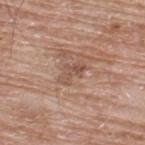workup: no biopsy performed (imaged during a skin exam) | subject: male, aged 63 to 67 | lesion size: ~3.5 mm (longest diameter) | image-analysis metrics: an outline eccentricity of about 0.85 (0 = round, 1 = elongated) and a symmetry-axis asymmetry near 0.4; an average lesion color of about L≈52 a*≈19 b*≈27 (CIELAB), roughly 8 lightness units darker than nearby skin, and a normalized border contrast of about 5.5; a within-lesion color-variation index near 2/10 and radial color variation of about 0.5; an automated nevus-likeness rating near 0 out of 100 and lesion-presence confidence of about 95/100 | image source: total-body-photography crop, ~15 mm field of view | anatomic site: the upper back.